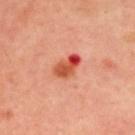The lesion was photographed on a routine skin check and not biopsied; there is no pathology result. The subject is a male about 50 years old. Automated tile analysis of the lesion measured border irregularity of about 2.5 on a 0–10 scale, internal color variation of about 10 on a 0–10 scale, and radial color variation of about 6. The lesion is on the upper back. A region of skin cropped from a whole-body photographic capture, roughly 15 mm wide. The recorded lesion diameter is about 4 mm.